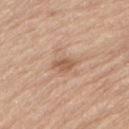biopsy status: no biopsy performed (imaged during a skin exam) | subject: male, approximately 70 years of age | image source: 15 mm crop, total-body photography | lesion size: about 3 mm | tile lighting: white-light | body site: the lower back.